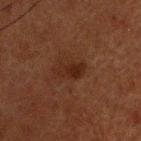Q: Was this lesion biopsied?
A: total-body-photography surveillance lesion; no biopsy
Q: What kind of image is this?
A: total-body-photography crop, ~15 mm field of view
Q: How large is the lesion?
A: ~3.5 mm (longest diameter)
Q: What are the patient's age and sex?
A: male, aged approximately 60
Q: Lesion location?
A: the head or neck
Q: Automated lesion metrics?
A: a footprint of about 5.5 mm², an outline eccentricity of about 0.8 (0 = round, 1 = elongated), and two-axis asymmetry of about 0.25; a mean CIELAB color near L≈19 a*≈17 b*≈22, about 5 CIELAB-L* units darker than the surrounding skin, and a lesion-to-skin contrast of about 7 (normalized; higher = more distinct); border irregularity of about 3 on a 0–10 scale and internal color variation of about 3 on a 0–10 scale; a classifier nevus-likeness of about 80/100 and lesion-presence confidence of about 100/100